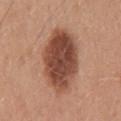acquisition=15 mm crop, total-body photography | anatomic site=the mid back | subject=male, aged 28 to 32.